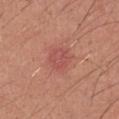workup: catalogued during a skin exam; not biopsied | lesion diameter: ≈3 mm | patient: male, aged around 35 | TBP lesion metrics: a shape eccentricity near 0.35; a border-irregularity index near 1.5/10 and a color-variation rating of about 3/10 | body site: the left upper arm | acquisition: ~15 mm tile from a whole-body skin photo.